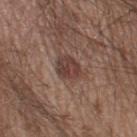workup = imaged on a skin check; not biopsied
acquisition = total-body-photography crop, ~15 mm field of view
patient = male, aged approximately 20
location = the left thigh